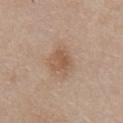<record>
  <biopsy_status>not biopsied; imaged during a skin examination</biopsy_status>
  <image>
    <source>total-body photography crop</source>
    <field_of_view_mm>15</field_of_view_mm>
  </image>
  <lesion_size>
    <long_diameter_mm_approx>4.0</long_diameter_mm_approx>
  </lesion_size>
  <site>chest</site>
  <automated_metrics>
    <area_mm2_approx>8.0</area_mm2_approx>
    <eccentricity>0.7</eccentricity>
    <shape_asymmetry>0.25</shape_asymmetry>
  </automated_metrics>
  <patient>
    <sex>male</sex>
    <age_approx>55</age_approx>
  </patient>
  <lighting>white-light</lighting>
</record>